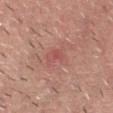This lesion was catalogued during total-body skin photography and was not selected for biopsy.
The subject is a male roughly 45 years of age.
Imaged with white-light lighting.
On the chest.
A roughly 15 mm field-of-view crop from a total-body skin photograph.
The lesion-visualizer software estimated a mean CIELAB color near L≈53 a*≈27 b*≈25 and about 6 CIELAB-L* units darker than the surrounding skin. And it measured a border-irregularity rating of about 4/10 and a color-variation rating of about 3/10.
The recorded lesion diameter is about 3.5 mm.